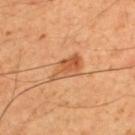<case>
  <biopsy_status>not biopsied; imaged during a skin examination</biopsy_status>
  <automated_metrics>
    <cielab_L>54</cielab_L>
    <cielab_a>25</cielab_a>
    <cielab_b>38</cielab_b>
    <vs_skin_darker_L>10.0</vs_skin_darker_L>
    <vs_skin_contrast_norm>7.0</vs_skin_contrast_norm>
    <nevus_likeness_0_100>55</nevus_likeness_0_100>
    <lesion_detection_confidence_0_100>100</lesion_detection_confidence_0_100>
  </automated_metrics>
  <patient>
    <sex>male</sex>
    <age_approx>50</age_approx>
  </patient>
  <lesion_size>
    <long_diameter_mm_approx>4.5</long_diameter_mm_approx>
  </lesion_size>
  <site>upper back</site>
  <lighting>cross-polarized</lighting>
  <image>
    <source>total-body photography crop</source>
    <field_of_view_mm>15</field_of_view_mm>
  </image>
</case>